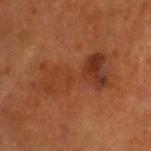A male subject aged approximately 60. Automated tile analysis of the lesion measured a classifier nevus-likeness of about 0/100 and a lesion-detection confidence of about 100/100. The lesion is on the head or neck. About 8 mm across. A region of skin cropped from a whole-body photographic capture, roughly 15 mm wide. Captured under cross-polarized illumination.